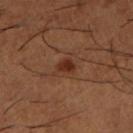Q: Is there a histopathology result?
A: no biopsy performed (imaged during a skin exam)
Q: What are the patient's age and sex?
A: male, in their mid- to late 60s
Q: What is the anatomic site?
A: the left lower leg
Q: How was the tile lit?
A: cross-polarized illumination
Q: Automated lesion metrics?
A: an eccentricity of roughly 0.65 and a symmetry-axis asymmetry near 0.3; a lesion color around L≈30 a*≈24 b*≈29 in CIELAB; border irregularity of about 2.5 on a 0–10 scale, a color-variation rating of about 1/10, and radial color variation of about 0.5
Q: How large is the lesion?
A: ≈2 mm
Q: How was this image acquired?
A: total-body-photography crop, ~15 mm field of view